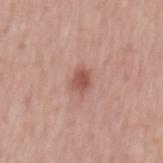Assessment: Imaged during a routine full-body skin examination; the lesion was not biopsied and no histopathology is available. Image and clinical context: Cropped from a total-body skin-imaging series; the visible field is about 15 mm. A male patient roughly 65 years of age. Measured at roughly 3 mm in maximum diameter. The lesion is located on the mid back.